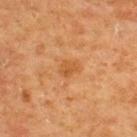Q: Was a biopsy performed?
A: catalogued during a skin exam; not biopsied
Q: Who is the patient?
A: male, about 65 years old
Q: What kind of image is this?
A: ~15 mm crop, total-body skin-cancer survey
Q: Where on the body is the lesion?
A: the upper back
Q: How large is the lesion?
A: ≈2.5 mm